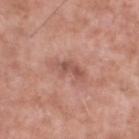workup: catalogued during a skin exam; not biopsied | automated lesion analysis: an area of roughly 5.5 mm² and an eccentricity of roughly 0.9; a mean CIELAB color near L≈54 a*≈24 b*≈26 and about 9 CIELAB-L* units darker than the surrounding skin; an automated nevus-likeness rating near 0 out of 100 and lesion-presence confidence of about 100/100 | location: the left lower leg | acquisition: ~15 mm crop, total-body skin-cancer survey | subject: male, aged 53 to 57 | diameter: ≈4 mm.